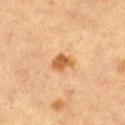  biopsy_status: not biopsied; imaged during a skin examination
  lesion_size:
    long_diameter_mm_approx: 2.5
  automated_metrics:
    cielab_L: 48
    cielab_a: 20
    cielab_b: 36
    vs_skin_darker_L: 12.0
    lesion_detection_confidence_0_100: 100
  image:
    source: total-body photography crop
    field_of_view_mm: 15
  patient:
    sex: female
    age_approx: 55
  site: left thigh
  lighting: cross-polarized